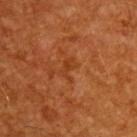Q: What is the lesion's diameter?
A: ≈2.5 mm
Q: Automated lesion metrics?
A: a border-irregularity index near 5/10 and internal color variation of about 1 on a 0–10 scale; a classifier nevus-likeness of about 0/100 and a detector confidence of about 100 out of 100 that the crop contains a lesion
Q: Lesion location?
A: the upper back
Q: What are the patient's age and sex?
A: male, aged 58 to 62
Q: How was the tile lit?
A: cross-polarized
Q: How was this image acquired?
A: 15 mm crop, total-body photography